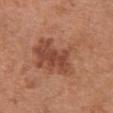| key | value |
|---|---|
| biopsy status | catalogued during a skin exam; not biopsied |
| tile lighting | white-light |
| anatomic site | the chest |
| imaging modality | ~15 mm tile from a whole-body skin photo |
| diameter | ~8 mm (longest diameter) |
| patient | female, aged around 65 |
| automated metrics | a footprint of about 28 mm²; an average lesion color of about L≈49 a*≈24 b*≈31 (CIELAB), a lesion–skin lightness drop of about 9, and a lesion-to-skin contrast of about 6.5 (normalized; higher = more distinct); a border-irregularity index near 5/10 |